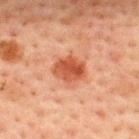biopsy status = no biopsy performed (imaged during a skin exam)
subject = male, roughly 60 years of age
lesion size = ~3.5 mm (longest diameter)
site = the upper back
acquisition = total-body-photography crop, ~15 mm field of view
TBP lesion metrics = a border-irregularity index near 1.5/10 and a color-variation rating of about 4.5/10
tile lighting = cross-polarized illumination